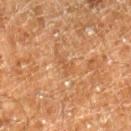follow-up: catalogued during a skin exam; not biopsied
size: about 2.5 mm
acquisition: total-body-photography crop, ~15 mm field of view
patient: male, approximately 60 years of age
automated metrics: a lesion color around L≈42 a*≈19 b*≈32 in CIELAB, roughly 5 lightness units darker than nearby skin, and a lesion-to-skin contrast of about 4.5 (normalized; higher = more distinct)
site: the right lower leg